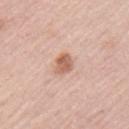{
  "biopsy_status": "not biopsied; imaged during a skin examination",
  "patient": {
    "sex": "male",
    "age_approx": 55
  },
  "lighting": "white-light",
  "site": "arm",
  "image": {
    "source": "total-body photography crop",
    "field_of_view_mm": 15
  },
  "automated_metrics": {
    "area_mm2_approx": 4.5,
    "eccentricity": 0.6,
    "shape_asymmetry": 0.2,
    "color_variation_0_10": 3.5,
    "peripheral_color_asymmetry": 1.5,
    "nevus_likeness_0_100": 85
  },
  "lesion_size": {
    "long_diameter_mm_approx": 2.5
  }
}